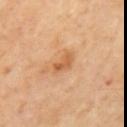{
  "image": {
    "source": "total-body photography crop",
    "field_of_view_mm": 15
  },
  "site": "mid back"
}